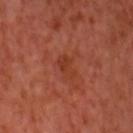<lesion>
<biopsy_status>not biopsied; imaged during a skin examination</biopsy_status>
</lesion>